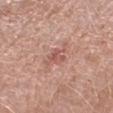Assessment:
The lesion was tiled from a total-body skin photograph and was not biopsied.
Image and clinical context:
About 4 mm across. A region of skin cropped from a whole-body photographic capture, roughly 15 mm wide. A female patient roughly 60 years of age. Automated tile analysis of the lesion measured a footprint of about 6 mm², an eccentricity of roughly 0.7, and a shape-asymmetry score of about 0.4 (0 = symmetric). The software also gave an average lesion color of about L≈56 a*≈24 b*≈26 (CIELAB), roughly 8 lightness units darker than nearby skin, and a normalized border contrast of about 5.5. The analysis additionally found a border-irregularity index near 5/10, a color-variation rating of about 3/10, and radial color variation of about 1. On the right forearm.